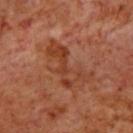No biopsy was performed on this lesion — it was imaged during a full skin examination and was not determined to be concerning. Approximately 6.5 mm at its widest. The lesion is located on the upper back. A male subject, in their 70s. A 15 mm close-up tile from a total-body photography series done for melanoma screening.